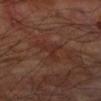Q: What are the patient's age and sex?
A: male, aged around 70
Q: How was the tile lit?
A: cross-polarized
Q: What is the imaging modality?
A: ~15 mm crop, total-body skin-cancer survey
Q: What is the anatomic site?
A: the left forearm
Q: How large is the lesion?
A: ≈4 mm
Q: What did automated image analysis measure?
A: about 5 CIELAB-L* units darker than the surrounding skin and a normalized lesion–skin contrast near 5